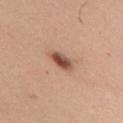Notes:
– follow-up: no biopsy performed (imaged during a skin exam)
– acquisition: total-body-photography crop, ~15 mm field of view
– illumination: white-light illumination
– lesion size: about 3 mm
– site: the upper back
– patient: male, about 55 years old